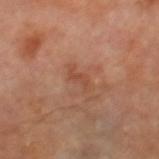Q: Was this lesion biopsied?
A: no biopsy performed (imaged during a skin exam)